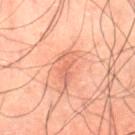Clinical impression: No biopsy was performed on this lesion — it was imaged during a full skin examination and was not determined to be concerning. Background: This image is a 15 mm lesion crop taken from a total-body photograph. Located on the abdomen. A male subject, aged approximately 50.